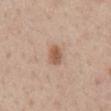Recorded during total-body skin imaging; not selected for excision or biopsy. Measured at roughly 3 mm in maximum diameter. A 15 mm close-up tile from a total-body photography series done for melanoma screening. Imaged with white-light lighting. From the mid back. A male patient in their mid-50s.